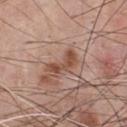No biopsy was performed on this lesion — it was imaged during a full skin examination and was not determined to be concerning.
The patient is a male aged 53–57.
The lesion is located on the chest.
A lesion tile, about 15 mm wide, cut from a 3D total-body photograph.
About 4 mm across.
Captured under white-light illumination.
The total-body-photography lesion software estimated an area of roughly 5.5 mm², a shape eccentricity near 0.9, and two-axis asymmetry of about 0.5. It also reported roughly 11 lightness units darker than nearby skin and a normalized border contrast of about 8. The software also gave a classifier nevus-likeness of about 15/100.